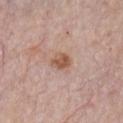The lesion was tiled from a total-body skin photograph and was not biopsied.